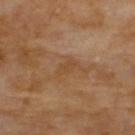– follow-up · imaged on a skin check; not biopsied
– subject · male, aged approximately 70
– acquisition · ~15 mm tile from a whole-body skin photo
– diameter · ≈2.5 mm
– body site · the upper back
– lighting · cross-polarized illumination